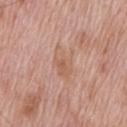biopsy_status: not biopsied; imaged during a skin examination
lesion_size:
  long_diameter_mm_approx: 4.0
lighting: white-light
site: chest
image:
  source: total-body photography crop
  field_of_view_mm: 15
patient:
  sex: male
  age_approx: 70